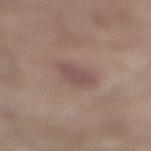Case summary:
* follow-up · total-body-photography surveillance lesion; no biopsy
* subject · male, aged approximately 80
* imaging modality · ~15 mm crop, total-body skin-cancer survey
* site · the right lower leg
* image-analysis metrics · a lesion area of about 5.5 mm², an eccentricity of roughly 0.65, and a symmetry-axis asymmetry near 0.2; a lesion color around L≈49 a*≈18 b*≈20 in CIELAB, a lesion–skin lightness drop of about 8, and a normalized border contrast of about 6
* diameter · ~3 mm (longest diameter)
* tile lighting · white-light